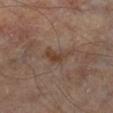Case summary:
• notes — catalogued during a skin exam; not biopsied
• size — ~4 mm (longest diameter)
• subject — male, aged 63 to 67
• location — the right lower leg
• automated metrics — an area of roughly 5 mm², an outline eccentricity of about 0.9 (0 = round, 1 = elongated), and a shape-asymmetry score of about 0.5 (0 = symmetric); about 7 CIELAB-L* units darker than the surrounding skin and a normalized lesion–skin contrast near 7; a classifier nevus-likeness of about 0/100 and lesion-presence confidence of about 100/100
• imaging modality — 15 mm crop, total-body photography